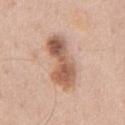workup: no biopsy performed (imaged during a skin exam)
imaging modality: 15 mm crop, total-body photography
illumination: white-light illumination
lesion size: about 6.5 mm
subject: male, aged around 60
TBP lesion metrics: a mean CIELAB color near L≈58 a*≈21 b*≈30 and about 14 CIELAB-L* units darker than the surrounding skin; a nevus-likeness score of about 55/100
anatomic site: the chest